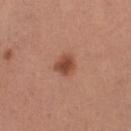Part of a total-body skin-imaging series; this lesion was reviewed on a skin check and was not flagged for biopsy. Located on the left thigh. A close-up tile cropped from a whole-body skin photograph, about 15 mm across. The subject is a female in their 30s. Captured under white-light illumination. The lesion-visualizer software estimated a lesion area of about 5 mm² and a symmetry-axis asymmetry near 0.25. The software also gave border irregularity of about 2 on a 0–10 scale, internal color variation of about 3 on a 0–10 scale, and radial color variation of about 1. And it measured an automated nevus-likeness rating near 95 out of 100 and a lesion-detection confidence of about 100/100. Longest diameter approximately 3 mm.